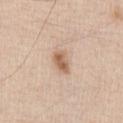- biopsy status — total-body-photography surveillance lesion; no biopsy
- body site — the chest
- subject — male, approximately 45 years of age
- lesion diameter — about 3 mm
- illumination — white-light illumination
- image — ~15 mm crop, total-body skin-cancer survey